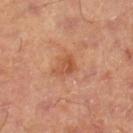This lesion was catalogued during total-body skin photography and was not selected for biopsy.